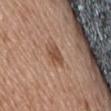The lesion was tiled from a total-body skin photograph and was not biopsied. The tile uses white-light illumination. A female subject, roughly 75 years of age. On the lower back. Longest diameter approximately 3 mm. Automated tile analysis of the lesion measured a footprint of about 5.5 mm², an eccentricity of roughly 0.7, and two-axis asymmetry of about 0.25. And it measured an average lesion color of about L≈50 a*≈19 b*≈29 (CIELAB) and roughly 10 lightness units darker than nearby skin. A roughly 15 mm field-of-view crop from a total-body skin photograph.